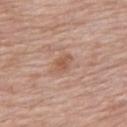Part of a total-body skin-imaging series; this lesion was reviewed on a skin check and was not flagged for biopsy. A lesion tile, about 15 mm wide, cut from a 3D total-body photograph. From the upper back. A female patient, about 70 years old.